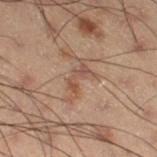Clinical impression:
The lesion was photographed on a routine skin check and not biopsied; there is no pathology result.
Background:
Cropped from a whole-body photographic skin survey; the tile spans about 15 mm. From the right thigh. A male subject in their mid-50s.